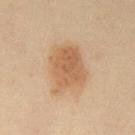follow-up: imaged on a skin check; not biopsied | lesion diameter: ≈6 mm | subject: male, about 50 years old | lighting: cross-polarized | image: ~15 mm tile from a whole-body skin photo | location: the chest.